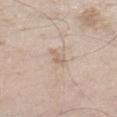| feature | finding |
|---|---|
| notes | no biopsy performed (imaged during a skin exam) |
| imaging modality | ~15 mm tile from a whole-body skin photo |
| patient | male, about 60 years old |
| location | the leg |